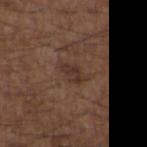Notes:
* notes — catalogued during a skin exam; not biopsied
* size — ~3 mm (longest diameter)
* imaging modality — ~15 mm crop, total-body skin-cancer survey
* tile lighting — white-light illumination
* subject — male, aged 48–52
* body site — the upper back